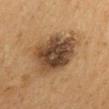Impression: Recorded during total-body skin imaging; not selected for excision or biopsy. Background: The lesion is located on the mid back. Cropped from a total-body skin-imaging series; the visible field is about 15 mm. The subject is a male aged 58 to 62. About 6.5 mm across. Captured under cross-polarized illumination.